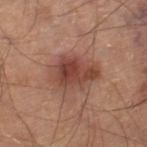<case>
<biopsy_status>not biopsied; imaged during a skin examination</biopsy_status>
<image>
  <source>total-body photography crop</source>
  <field_of_view_mm>15</field_of_view_mm>
</image>
<lesion_size>
  <long_diameter_mm_approx>5.0</long_diameter_mm_approx>
</lesion_size>
<site>right thigh</site>
<automated_metrics>
  <eccentricity>0.7</eccentricity>
  <shape_asymmetry>0.2</shape_asymmetry>
  <cielab_L>45</cielab_L>
  <cielab_a>24</cielab_a>
  <cielab_b>27</cielab_b>
  <vs_skin_darker_L>11.0</vs_skin_darker_L>
  <nevus_likeness_0_100>95</nevus_likeness_0_100>
  <lesion_detection_confidence_0_100>100</lesion_detection_confidence_0_100>
</automated_metrics>
<lighting>cross-polarized</lighting>
</case>